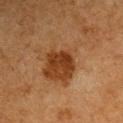Imaged during a routine full-body skin examination; the lesion was not biopsied and no histopathology is available.
The subject is a male aged around 65.
The lesion is on the right upper arm.
Imaged with cross-polarized lighting.
Automated tile analysis of the lesion measured an average lesion color of about L≈31 a*≈23 b*≈33 (CIELAB), a lesion–skin lightness drop of about 10, and a lesion-to-skin contrast of about 9.5 (normalized; higher = more distinct). And it measured a border-irregularity rating of about 4.5/10, a color-variation rating of about 2/10, and a peripheral color-asymmetry measure near 1. The analysis additionally found a lesion-detection confidence of about 100/100.
The recorded lesion diameter is about 3 mm.
A region of skin cropped from a whole-body photographic capture, roughly 15 mm wide.